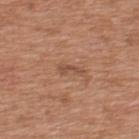Clinical impression:
The lesion was photographed on a routine skin check and not biopsied; there is no pathology result.
Image and clinical context:
Captured under white-light illumination. About 2.5 mm across. The lesion is located on the upper back. An algorithmic analysis of the crop reported a lesion area of about 2.5 mm², a shape eccentricity near 0.9, and a symmetry-axis asymmetry near 0.5. The software also gave a mean CIELAB color near L≈49 a*≈22 b*≈31. The analysis additionally found a nevus-likeness score of about 0/100 and lesion-presence confidence of about 100/100. A male patient aged around 65. A lesion tile, about 15 mm wide, cut from a 3D total-body photograph.